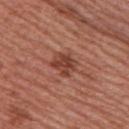| field | value |
|---|---|
| biopsy status | imaged on a skin check; not biopsied |
| TBP lesion metrics | a mean CIELAB color near L≈43 a*≈26 b*≈29 and about 10 CIELAB-L* units darker than the surrounding skin; a classifier nevus-likeness of about 75/100 and a detector confidence of about 100 out of 100 that the crop contains a lesion |
| diameter | ~3 mm (longest diameter) |
| imaging modality | ~15 mm crop, total-body skin-cancer survey |
| illumination | white-light |
| subject | female, in their mid-50s |
| location | the upper back |